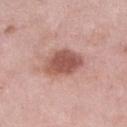– imaging modality — ~15 mm tile from a whole-body skin photo
– anatomic site — the right lower leg
– illumination — white-light
– subject — female, approximately 70 years of age
– lesion size — ~4.5 mm (longest diameter)